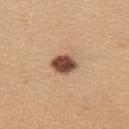The lesion was tiled from a total-body skin photograph and was not biopsied. Longest diameter approximately 3.5 mm. A 15 mm close-up tile from a total-body photography series done for melanoma screening. From the upper back. A female patient, aged approximately 45. Automated tile analysis of the lesion measured border irregularity of about 1 on a 0–10 scale, internal color variation of about 3.5 on a 0–10 scale, and peripheral color asymmetry of about 1.